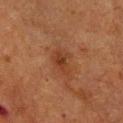Recorded during total-body skin imaging; not selected for excision or biopsy.